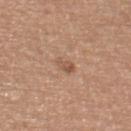{"site": "back", "image": {"source": "total-body photography crop", "field_of_view_mm": 15}, "patient": {"sex": "male", "age_approx": 65}, "lesion_size": {"long_diameter_mm_approx": 2.5}}